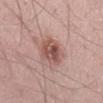Assessment: This lesion was catalogued during total-body skin photography and was not selected for biopsy. Acquisition and patient details: Captured under white-light illumination. Cropped from a whole-body photographic skin survey; the tile spans about 15 mm. From the left thigh. Measured at roughly 4.5 mm in maximum diameter. A male patient aged 53 to 57.